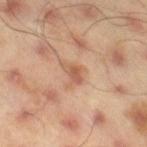The tile uses cross-polarized illumination. Located on the right thigh. A male patient, aged 43–47. A roughly 15 mm field-of-view crop from a total-body skin photograph. Longest diameter approximately 3 mm.